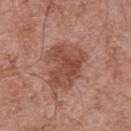notes: no biopsy performed (imaged during a skin exam) | image-analysis metrics: an area of roughly 19 mm² and a shape eccentricity near 0.65; an average lesion color of about L≈48 a*≈24 b*≈29 (CIELAB), roughly 10 lightness units darker than nearby skin, and a lesion-to-skin contrast of about 7.5 (normalized; higher = more distinct) | site: the chest | imaging modality: ~15 mm crop, total-body skin-cancer survey | lighting: white-light | lesion size: about 5.5 mm | subject: male, approximately 70 years of age.